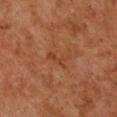The lesion was tiled from a total-body skin photograph and was not biopsied. A close-up tile cropped from a whole-body skin photograph, about 15 mm across. A female patient aged 58–62. The tile uses cross-polarized illumination. From the chest.